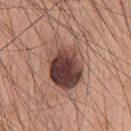The lesion was photographed on a routine skin check and not biopsied; there is no pathology result. The tile uses white-light illumination. The recorded lesion diameter is about 6.5 mm. A 15 mm close-up extracted from a 3D total-body photography capture. The lesion-visualizer software estimated a border-irregularity rating of about 2.5/10 and a color-variation rating of about 10/10. The analysis additionally found an automated nevus-likeness rating near 55 out of 100 and a lesion-detection confidence of about 100/100. Located on the chest. A male subject aged 58–62.